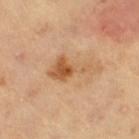Clinical impression:
Recorded during total-body skin imaging; not selected for excision or biopsy.
Context:
Measured at roughly 5.5 mm in maximum diameter. The lesion is on the right thigh. A 15 mm crop from a total-body photograph taken for skin-cancer surveillance. Imaged with cross-polarized lighting. A female patient aged 68–72.